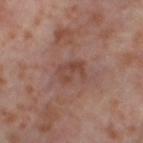Notes:
• biopsy status: total-body-photography surveillance lesion; no biopsy
• illumination: cross-polarized illumination
• automated metrics: a classifier nevus-likeness of about 0/100 and lesion-presence confidence of about 100/100
• lesion size: ≈3.5 mm
• patient: female, aged 53–57
• site: the left thigh
• image: ~15 mm tile from a whole-body skin photo